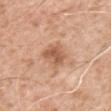Clinical impression: No biopsy was performed on this lesion — it was imaged during a full skin examination and was not determined to be concerning. Clinical summary: Located on the chest. Measured at roughly 3 mm in maximum diameter. Imaged with white-light lighting. A region of skin cropped from a whole-body photographic capture, roughly 15 mm wide. The patient is a male aged around 60. The total-body-photography lesion software estimated a lesion color around L≈58 a*≈22 b*≈33 in CIELAB and about 11 CIELAB-L* units darker than the surrounding skin. The analysis additionally found a border-irregularity index near 2/10, a color-variation rating of about 4/10, and a peripheral color-asymmetry measure near 1.5. It also reported an automated nevus-likeness rating near 15 out of 100 and a detector confidence of about 100 out of 100 that the crop contains a lesion.